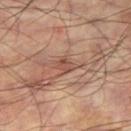Clinical impression:
Imaged during a routine full-body skin examination; the lesion was not biopsied and no histopathology is available.
Background:
A male subject roughly 60 years of age. Approximately 3 mm at its widest. The tile uses cross-polarized illumination. The lesion is located on the right thigh. A 15 mm crop from a total-body photograph taken for skin-cancer surveillance.